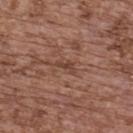diameter: ~2.5 mm (longest diameter)
tile lighting: white-light
anatomic site: the upper back
image-analysis metrics: a lesion color around L≈42 a*≈21 b*≈27 in CIELAB and a normalized border contrast of about 6.5; a border-irregularity rating of about 4/10, a within-lesion color-variation index near 0/10, and a peripheral color-asymmetry measure near 0
image: 15 mm crop, total-body photography
patient: female, in their mid- to late 60s